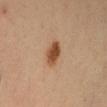Case summary:
• follow-up — no biopsy performed (imaged during a skin exam)
• tile lighting — cross-polarized illumination
• location — the chest
• size — ≈3.5 mm
• acquisition — ~15 mm tile from a whole-body skin photo
• subject — male, about 35 years old